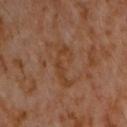The lesion was photographed on a routine skin check and not biopsied; there is no pathology result. Imaged with cross-polarized lighting. Automated image analysis of the tile measured a color-variation rating of about 1.5/10. The patient is a male aged 58 to 62. From the chest. A roughly 15 mm field-of-view crop from a total-body skin photograph.